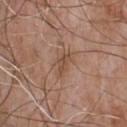Part of a total-body skin-imaging series; this lesion was reviewed on a skin check and was not flagged for biopsy.
The total-body-photography lesion software estimated a footprint of about 5 mm², a shape eccentricity near 0.75, and a shape-asymmetry score of about 0.4 (0 = symmetric). The software also gave a lesion color around L≈49 a*≈19 b*≈29 in CIELAB, a lesion–skin lightness drop of about 8, and a normalized border contrast of about 6. The software also gave border irregularity of about 4.5 on a 0–10 scale, a within-lesion color-variation index near 3/10, and radial color variation of about 1. The software also gave a nevus-likeness score of about 0/100.
A roughly 15 mm field-of-view crop from a total-body skin photograph.
The lesion is located on the chest.
The patient is a male approximately 65 years of age.